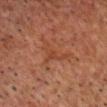Clinical impression:
Recorded during total-body skin imaging; not selected for excision or biopsy.
Context:
Measured at roughly 4 mm in maximum diameter. Automated image analysis of the tile measured roughly 5 lightness units darker than nearby skin and a normalized border contrast of about 5. And it measured border irregularity of about 9.5 on a 0–10 scale, internal color variation of about 1 on a 0–10 scale, and radial color variation of about 0.5. The lesion is located on the head or neck. A male subject, aged around 60. Imaged with cross-polarized lighting. A region of skin cropped from a whole-body photographic capture, roughly 15 mm wide.